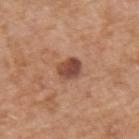workup — total-body-photography surveillance lesion; no biopsy
TBP lesion metrics — a lesion color around L≈47 a*≈23 b*≈29 in CIELAB, a lesion–skin lightness drop of about 13, and a lesion-to-skin contrast of about 10 (normalized; higher = more distinct); a border-irregularity index near 1/10, a color-variation rating of about 4/10, and peripheral color asymmetry of about 1.5
lesion size — ~3 mm (longest diameter)
patient — male, aged 58 to 62
imaging modality — 15 mm crop, total-body photography
anatomic site — the upper back